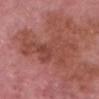The lesion was tiled from a total-body skin photograph and was not biopsied.